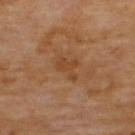Clinical summary:
Located on the back. A male patient, aged approximately 70. A roughly 15 mm field-of-view crop from a total-body skin photograph. Automated tile analysis of the lesion measured a shape eccentricity near 0.75. It also reported an average lesion color of about L≈43 a*≈21 b*≈35 (CIELAB) and a normalized border contrast of about 5.5. Measured at roughly 3.5 mm in maximum diameter. Captured under cross-polarized illumination.